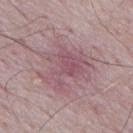| feature | finding |
|---|---|
| follow-up | catalogued during a skin exam; not biopsied |
| subject | male, aged approximately 65 |
| body site | the mid back |
| acquisition | ~15 mm tile from a whole-body skin photo |
| automated metrics | an average lesion color of about L≈53 a*≈22 b*≈14 (CIELAB) and a lesion–skin lightness drop of about 8; a border-irregularity index near 7.5/10, internal color variation of about 3.5 on a 0–10 scale, and radial color variation of about 1; a nevus-likeness score of about 0/100 |
| diameter | about 6 mm |
| illumination | white-light |